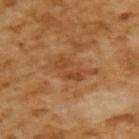This lesion was catalogued during total-body skin photography and was not selected for biopsy. A male patient aged approximately 65. Captured under cross-polarized illumination. Longest diameter approximately 3.5 mm. A roughly 15 mm field-of-view crop from a total-body skin photograph.